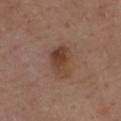This is a white-light tile. The lesion is on the upper back. This image is a 15 mm lesion crop taken from a total-body photograph. Approximately 4.5 mm at its widest. A male subject aged 68–72. Automated image analysis of the tile measured a shape eccentricity near 0.8. And it measured a border-irregularity index near 2.5/10, a within-lesion color-variation index near 6/10, and radial color variation of about 2.5. The software also gave a classifier nevus-likeness of about 65/100 and lesion-presence confidence of about 100/100.